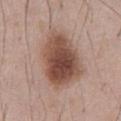No biopsy was performed on this lesion — it was imaged during a full skin examination and was not determined to be concerning. A 15 mm close-up tile from a total-body photography series done for melanoma screening. The subject is a male in their mid-50s. The lesion is on the abdomen.